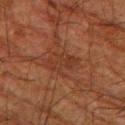Clinical impression:
Imaged during a routine full-body skin examination; the lesion was not biopsied and no histopathology is available.
Clinical summary:
A 15 mm close-up extracted from a 3D total-body photography capture. The tile uses cross-polarized illumination. The lesion is on the left thigh. The lesion's longest dimension is about 4 mm. The patient is a male roughly 80 years of age.